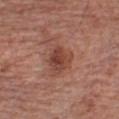Captured during whole-body skin photography for melanoma surveillance; the lesion was not biopsied. Longest diameter approximately 4 mm. A region of skin cropped from a whole-body photographic capture, roughly 15 mm wide. An algorithmic analysis of the crop reported a detector confidence of about 100 out of 100 that the crop contains a lesion. A male patient, roughly 65 years of age. On the chest. Imaged with white-light lighting.